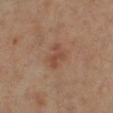Impression: Part of a total-body skin-imaging series; this lesion was reviewed on a skin check and was not flagged for biopsy. Acquisition and patient details: A 15 mm crop from a total-body photograph taken for skin-cancer surveillance. On the left lower leg. A male subject, in their 50s.